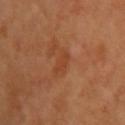follow-up: catalogued during a skin exam; not biopsied | imaging modality: ~15 mm crop, total-body skin-cancer survey | location: the arm | image-analysis metrics: a footprint of about 3 mm² and a shape-asymmetry score of about 0.45 (0 = symmetric); a lesion color around L≈43 a*≈27 b*≈37 in CIELAB, about 6 CIELAB-L* units darker than the surrounding skin, and a normalized lesion–skin contrast near 5; border irregularity of about 4 on a 0–10 scale, internal color variation of about 1 on a 0–10 scale, and a peripheral color-asymmetry measure near 0.5; an automated nevus-likeness rating near 0 out of 100 | patient: female, aged 58 to 62 | size: ~2.5 mm (longest diameter).